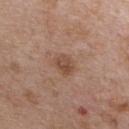Automated tile analysis of the lesion measured an area of roughly 4 mm², an eccentricity of roughly 0.7, and two-axis asymmetry of about 0.3. A close-up tile cropped from a whole-body skin photograph, about 15 mm across. This is a white-light tile. About 2.5 mm across. From the chest. The patient is a male aged around 65.